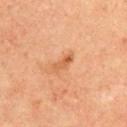A region of skin cropped from a whole-body photographic capture, roughly 15 mm wide.
From the front of the torso.
The subject is a female about 55 years old.
The total-body-photography lesion software estimated a border-irregularity rating of about 3/10. The analysis additionally found a nevus-likeness score of about 15/100 and a lesion-detection confidence of about 100/100.
Longest diameter approximately 3 mm.
The tile uses cross-polarized illumination.